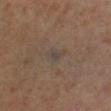Assessment: No biopsy was performed on this lesion — it was imaged during a full skin examination and was not determined to be concerning. Acquisition and patient details: A female patient, in their 50s. This is a cross-polarized tile. The recorded lesion diameter is about 3 mm. On the left lower leg. A 15 mm close-up extracted from a 3D total-body photography capture.